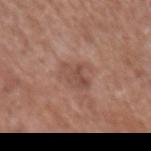follow-up — no biopsy performed (imaged during a skin exam) | automated lesion analysis — a lesion area of about 6.5 mm², an outline eccentricity of about 0.45 (0 = round, 1 = elongated), and a shape-asymmetry score of about 0.35 (0 = symmetric); a border-irregularity index near 4/10, a color-variation rating of about 3.5/10, and radial color variation of about 1.5 | tile lighting — white-light illumination | anatomic site — the left upper arm | subject — male, aged 68 to 72 | acquisition — ~15 mm tile from a whole-body skin photo | size — ~3 mm (longest diameter).